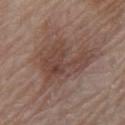Q: Is there a histopathology result?
A: catalogued during a skin exam; not biopsied
Q: Who is the patient?
A: male, in their 80s
Q: What is the imaging modality?
A: ~15 mm crop, total-body skin-cancer survey
Q: What did automated image analysis measure?
A: an average lesion color of about L≈44 a*≈18 b*≈23 (CIELAB), roughly 8 lightness units darker than nearby skin, and a normalized border contrast of about 6.5; an automated nevus-likeness rating near 0 out of 100 and lesion-presence confidence of about 100/100
Q: How large is the lesion?
A: ≈7.5 mm
Q: What is the anatomic site?
A: the arm
Q: What lighting was used for the tile?
A: white-light